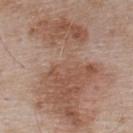Part of a total-body skin-imaging series; this lesion was reviewed on a skin check and was not flagged for biopsy. The tile uses white-light illumination. A lesion tile, about 15 mm wide, cut from a 3D total-body photograph. From the upper back. A male patient, aged 53 to 57.